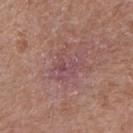Automated tile analysis of the lesion measured a lesion area of about 8.5 mm² and an eccentricity of roughly 0.45. The analysis additionally found roughly 5 lightness units darker than nearby skin and a lesion-to-skin contrast of about 5.5 (normalized; higher = more distinct). The analysis additionally found a classifier nevus-likeness of about 0/100 and a lesion-detection confidence of about 95/100. Captured under white-light illumination. The recorded lesion diameter is about 4 mm. A close-up tile cropped from a whole-body skin photograph, about 15 mm across. From the left arm. A male subject, roughly 80 years of age.